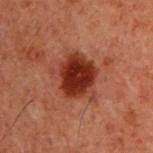workup — catalogued during a skin exam; not biopsied | site — the upper back | diameter — about 5.5 mm | automated lesion analysis — a lesion area of about 17 mm² and a shape eccentricity near 0.45; an average lesion color of about L≈26 a*≈24 b*≈26 (CIELAB); a border-irregularity index near 3.5/10, internal color variation of about 4 on a 0–10 scale, and a peripheral color-asymmetry measure near 1 | subject — male, aged 58–62 | tile lighting — cross-polarized illumination | acquisition — ~15 mm tile from a whole-body skin photo.